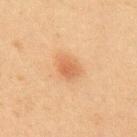Captured during whole-body skin photography for melanoma surveillance; the lesion was not biopsied. An algorithmic analysis of the crop reported an area of roughly 4.5 mm², an outline eccentricity of about 0.6 (0 = round, 1 = elongated), and two-axis asymmetry of about 0.25. The software also gave an automated nevus-likeness rating near 95 out of 100 and a detector confidence of about 100 out of 100 that the crop contains a lesion. A close-up tile cropped from a whole-body skin photograph, about 15 mm across. From the upper back. A male subject aged 43–47.